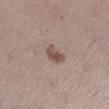location — the left lower leg | image source — ~15 mm crop, total-body skin-cancer survey | subject — female, aged 28 to 32 | automated lesion analysis — a border-irregularity index near 3/10 and radial color variation of about 1.5; a classifier nevus-likeness of about 70/100 and a detector confidence of about 100 out of 100 that the crop contains a lesion | tile lighting — white-light illumination.